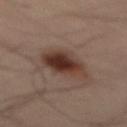No biopsy was performed on this lesion — it was imaged during a full skin examination and was not determined to be concerning.
Located on the mid back.
Automated image analysis of the tile measured roughly 13 lightness units darker than nearby skin and a normalized lesion–skin contrast near 11.5. It also reported border irregularity of about 2.5 on a 0–10 scale and a within-lesion color-variation index near 6.5/10.
The tile uses cross-polarized illumination.
A region of skin cropped from a whole-body photographic capture, roughly 15 mm wide.
The subject is a male approximately 50 years of age.